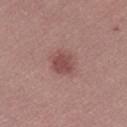Captured under white-light illumination. The recorded lesion diameter is about 2.5 mm. The subject is a female in their mid- to late 30s. The lesion is on the left leg. A 15 mm close-up extracted from a 3D total-body photography capture.